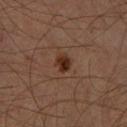Part of a total-body skin-imaging series; this lesion was reviewed on a skin check and was not flagged for biopsy.
The lesion is on the right lower leg.
A 15 mm close-up extracted from a 3D total-body photography capture.
The total-body-photography lesion software estimated a symmetry-axis asymmetry near 0.3. It also reported roughly 10 lightness units darker than nearby skin and a normalized lesion–skin contrast near 10. The analysis additionally found a border-irregularity index near 2.5/10, a within-lesion color-variation index near 4/10, and a peripheral color-asymmetry measure near 1.5. It also reported an automated nevus-likeness rating near 95 out of 100 and lesion-presence confidence of about 100/100.
A male patient in their 60s.
This is a cross-polarized tile.